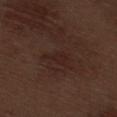| feature | finding |
|---|---|
| notes | no biopsy performed (imaged during a skin exam) |
| illumination | white-light |
| image source | ~15 mm crop, total-body skin-cancer survey |
| location | the right thigh |
| diameter | about 3 mm |
| patient | male, aged 68–72 |